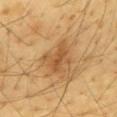Impression: Part of a total-body skin-imaging series; this lesion was reviewed on a skin check and was not flagged for biopsy.